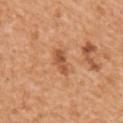Captured during whole-body skin photography for melanoma surveillance; the lesion was not biopsied.
The total-body-photography lesion software estimated a border-irregularity rating of about 3.5/10, a color-variation rating of about 1.5/10, and peripheral color asymmetry of about 0.5. The analysis additionally found lesion-presence confidence of about 100/100.
Imaged with white-light lighting.
Measured at roughly 3.5 mm in maximum diameter.
From the right upper arm.
Cropped from a whole-body photographic skin survey; the tile spans about 15 mm.
A male patient, in their mid- to late 50s.